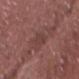workup — no biopsy performed (imaged during a skin exam)
image source — ~15 mm tile from a whole-body skin photo
lighting — white-light
image-analysis metrics — a lesion area of about 5 mm² and an outline eccentricity of about 0.85 (0 = round, 1 = elongated); a border-irregularity index near 2.5/10 and radial color variation of about 0.5
body site — the chest
patient — male, about 70 years old